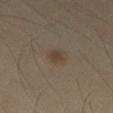Recorded during total-body skin imaging; not selected for excision or biopsy. An algorithmic analysis of the crop reported roughly 7 lightness units darker than nearby skin and a lesion-to-skin contrast of about 7 (normalized; higher = more distinct). The analysis additionally found a border-irregularity rating of about 2/10, internal color variation of about 1.5 on a 0–10 scale, and radial color variation of about 0.5. It also reported an automated nevus-likeness rating near 85 out of 100 and lesion-presence confidence of about 100/100. Cropped from a total-body skin-imaging series; the visible field is about 15 mm. Approximately 2.5 mm at its widest. The patient is a male aged approximately 30. The lesion is on the left thigh. Captured under cross-polarized illumination.